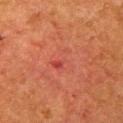Assessment:
This lesion was catalogued during total-body skin photography and was not selected for biopsy.
Background:
This is a cross-polarized tile. Longest diameter approximately 3 mm. A female patient, aged approximately 50. On the arm. A lesion tile, about 15 mm wide, cut from a 3D total-body photograph.